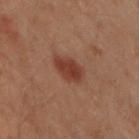Part of a total-body skin-imaging series; this lesion was reviewed on a skin check and was not flagged for biopsy. A male patient about 30 years old. Longest diameter approximately 3 mm. This image is a 15 mm lesion crop taken from a total-body photograph. Captured under cross-polarized illumination. The lesion-visualizer software estimated a footprint of about 7 mm², a shape eccentricity near 0.65, and two-axis asymmetry of about 0.2. It also reported a mean CIELAB color near L≈30 a*≈20 b*≈24 and about 8 CIELAB-L* units darker than the surrounding skin. It also reported a border-irregularity rating of about 2/10, a within-lesion color-variation index near 2/10, and a peripheral color-asymmetry measure near 0.5. The lesion is on the upper back.